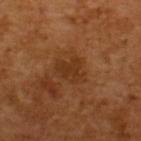notes — no biopsy performed (imaged during a skin exam)
image source — 15 mm crop, total-body photography
TBP lesion metrics — a mean CIELAB color near L≈33 a*≈22 b*≈34, roughly 6 lightness units darker than nearby skin, and a lesion-to-skin contrast of about 6 (normalized; higher = more distinct); a border-irregularity index near 5/10, a color-variation rating of about 2/10, and peripheral color asymmetry of about 0.5
illumination — cross-polarized illumination
subject — male, about 65 years old